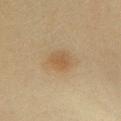Part of a total-body skin-imaging series; this lesion was reviewed on a skin check and was not flagged for biopsy.
Cropped from a total-body skin-imaging series; the visible field is about 15 mm.
The tile uses cross-polarized illumination.
A female subject roughly 50 years of age.
The lesion is on the left lower leg.
Approximately 3 mm at its widest.